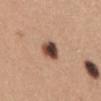Impression: Part of a total-body skin-imaging series; this lesion was reviewed on a skin check and was not flagged for biopsy. Image and clinical context: The lesion is located on the abdomen. The recorded lesion diameter is about 3 mm. A female subject about 30 years old. Captured under white-light illumination. A 15 mm crop from a total-body photograph taken for skin-cancer surveillance.